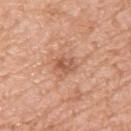Findings:
* workup: imaged on a skin check; not biopsied
* TBP lesion metrics: roughly 10 lightness units darker than nearby skin and a lesion-to-skin contrast of about 6.5 (normalized; higher = more distinct); a nevus-likeness score of about 0/100 and lesion-presence confidence of about 100/100
* patient: female, roughly 55 years of age
* imaging modality: total-body-photography crop, ~15 mm field of view
* lesion size: ~2.5 mm (longest diameter)
* location: the left upper arm
* tile lighting: white-light